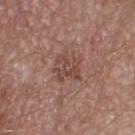Q: Was this lesion biopsied?
A: imaged on a skin check; not biopsied
Q: Patient demographics?
A: male, in their mid- to late 50s
Q: What kind of image is this?
A: ~15 mm crop, total-body skin-cancer survey
Q: Where on the body is the lesion?
A: the right thigh
Q: How large is the lesion?
A: about 3.5 mm
Q: Illumination type?
A: white-light illumination